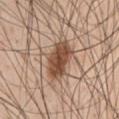| feature | finding |
|---|---|
| follow-up | no biopsy performed (imaged during a skin exam) |
| patient | male, aged 58 to 62 |
| site | the chest |
| tile lighting | white-light |
| image source | ~15 mm tile from a whole-body skin photo |